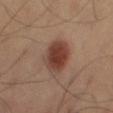Part of a total-body skin-imaging series; this lesion was reviewed on a skin check and was not flagged for biopsy.
The lesion is on the right thigh.
A male subject roughly 60 years of age.
A close-up tile cropped from a whole-body skin photograph, about 15 mm across.